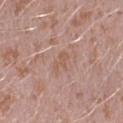Recorded during total-body skin imaging; not selected for excision or biopsy. This image is a 15 mm lesion crop taken from a total-body photograph. This is a white-light tile. Longest diameter approximately 3 mm. The patient is a male approximately 40 years of age. Located on the left forearm.